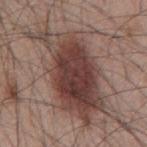Impression:
No biopsy was performed on this lesion — it was imaged during a full skin examination and was not determined to be concerning.
Clinical summary:
A male patient about 45 years old. Measured at roughly 12 mm in maximum diameter. Automated image analysis of the tile measured peripheral color asymmetry of about 2.5. And it measured an automated nevus-likeness rating near 95 out of 100 and a detector confidence of about 100 out of 100 that the crop contains a lesion. On the mid back. A lesion tile, about 15 mm wide, cut from a 3D total-body photograph. Imaged with white-light lighting.